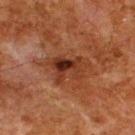No biopsy was performed on this lesion — it was imaged during a full skin examination and was not determined to be concerning. A 15 mm close-up tile from a total-body photography series done for melanoma screening. From the upper back. Approximately 4 mm at its widest. A male subject, approximately 80 years of age.